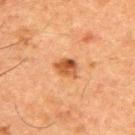{"biopsy_status": "not biopsied; imaged during a skin examination", "image": {"source": "total-body photography crop", "field_of_view_mm": 15}, "lighting": "cross-polarized", "patient": {"sex": "male", "age_approx": 50}, "site": "upper back", "lesion_size": {"long_diameter_mm_approx": 3.0}}